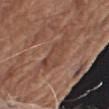Clinical impression:
Imaged during a routine full-body skin examination; the lesion was not biopsied and no histopathology is available.
Background:
The subject is a male aged approximately 75. A lesion tile, about 15 mm wide, cut from a 3D total-body photograph. This is a white-light tile. On the right upper arm.